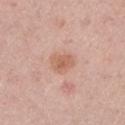Recorded during total-body skin imaging; not selected for excision or biopsy.
This image is a 15 mm lesion crop taken from a total-body photograph.
Approximately 3 mm at its widest.
The tile uses white-light illumination.
From the left upper arm.
The patient is a male aged 43 to 47.